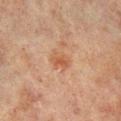Part of a total-body skin-imaging series; this lesion was reviewed on a skin check and was not flagged for biopsy.
This is a cross-polarized tile.
Approximately 2.5 mm at its widest.
A 15 mm close-up extracted from a 3D total-body photography capture.
The lesion is on the right lower leg.
A male subject, aged 68–72.